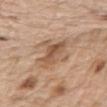Recorded during total-body skin imaging; not selected for excision or biopsy.
A roughly 15 mm field-of-view crop from a total-body skin photograph.
Captured under white-light illumination.
The lesion is on the arm.
A male patient, about 70 years old.